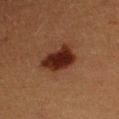Assessment: The lesion was photographed on a routine skin check and not biopsied; there is no pathology result. Clinical summary: The lesion is on the left upper arm. Cropped from a total-body skin-imaging series; the visible field is about 15 mm. The subject is a male aged around 40.